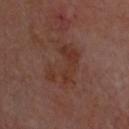| field | value |
|---|---|
| notes | total-body-photography surveillance lesion; no biopsy |